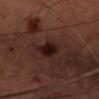Impression:
This lesion was catalogued during total-body skin photography and was not selected for biopsy.
Context:
Located on the head or neck. A female subject, aged 43 to 47. A 15 mm close-up tile from a total-body photography series done for melanoma screening.